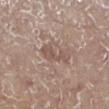The lesion was tiled from a total-body skin photograph and was not biopsied. Imaged with white-light lighting. The lesion's longest dimension is about 4 mm. A close-up tile cropped from a whole-body skin photograph, about 15 mm across. From the left lower leg. The total-body-photography lesion software estimated a symmetry-axis asymmetry near 0.4. It also reported a normalized border contrast of about 5.5. And it measured a nevus-likeness score of about 0/100 and a lesion-detection confidence of about 100/100. A male patient aged approximately 70.